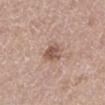biopsy status=no biopsy performed (imaged during a skin exam); body site=the leg; acquisition=total-body-photography crop, ~15 mm field of view; patient=female, aged 48–52.